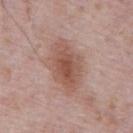| field | value |
|---|---|
| workup | catalogued during a skin exam; not biopsied |
| patient | male, in their 70s |
| location | the abdomen |
| automated lesion analysis | a border-irregularity rating of about 2/10, a color-variation rating of about 4.5/10, and radial color variation of about 1 |
| lesion size | ~6.5 mm (longest diameter) |
| acquisition | 15 mm crop, total-body photography |
| illumination | white-light illumination |